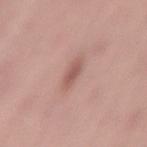This lesion was catalogued during total-body skin photography and was not selected for biopsy. The lesion is on the left thigh. A male patient aged 53–57. A 15 mm crop from a total-body photograph taken for skin-cancer surveillance.